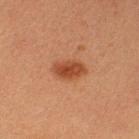{"site": "left thigh", "image": {"source": "total-body photography crop", "field_of_view_mm": 15}, "patient": {"sex": "female", "age_approx": 40}}